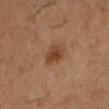biopsy_status: not biopsied; imaged during a skin examination
patient:
  sex: female
  age_approx: 50
lesion_size:
  long_diameter_mm_approx: 3.0
image:
  source: total-body photography crop
  field_of_view_mm: 15
site: left lower leg
lighting: cross-polarized
automated_metrics:
  area_mm2_approx: 6.0
  eccentricity: 0.7
  shape_asymmetry: 0.2